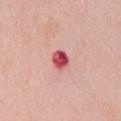A female patient about 50 years old. A 15 mm close-up extracted from a 3D total-body photography capture. The lesion is on the mid back. Measured at roughly 2.5 mm in maximum diameter.